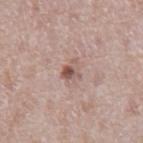The lesion was photographed on a routine skin check and not biopsied; there is no pathology result. The lesion is located on the abdomen. An algorithmic analysis of the crop reported a footprint of about 4 mm² and two-axis asymmetry of about 0.35. The analysis additionally found a border-irregularity index near 3.5/10, internal color variation of about 7 on a 0–10 scale, and peripheral color asymmetry of about 2. It also reported an automated nevus-likeness rating near 40 out of 100. A male patient approximately 70 years of age. Cropped from a whole-body photographic skin survey; the tile spans about 15 mm. Imaged with white-light lighting.